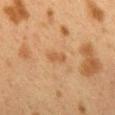Q: Was a biopsy performed?
A: catalogued during a skin exam; not biopsied
Q: What kind of image is this?
A: total-body-photography crop, ~15 mm field of view
Q: Who is the patient?
A: female, roughly 40 years of age
Q: Where on the body is the lesion?
A: the mid back
Q: How was the tile lit?
A: cross-polarized